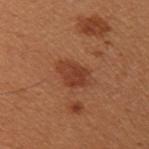This lesion was catalogued during total-body skin photography and was not selected for biopsy. A 15 mm close-up extracted from a 3D total-body photography capture. Automated image analysis of the tile measured a border-irregularity rating of about 2.5/10, internal color variation of about 2.5 on a 0–10 scale, and a peripheral color-asymmetry measure near 1. It also reported a classifier nevus-likeness of about 35/100. About 3.5 mm across. The lesion is located on the left upper arm. A female patient, in their 50s.